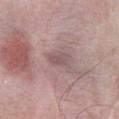Clinical impression:
Imaged during a routine full-body skin examination; the lesion was not biopsied and no histopathology is available.
Background:
On the abdomen. The recorded lesion diameter is about 3 mm. The total-body-photography lesion software estimated an average lesion color of about L≈53 a*≈18 b*≈17 (CIELAB) and a lesion-to-skin contrast of about 5.5 (normalized; higher = more distinct). The analysis additionally found a border-irregularity index near 2/10, a color-variation rating of about 2/10, and a peripheral color-asymmetry measure near 0.5. The patient is a male aged 58 to 62. A region of skin cropped from a whole-body photographic capture, roughly 15 mm wide.